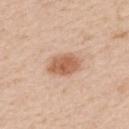workup=imaged on a skin check; not biopsied | location=the upper back | image=~15 mm tile from a whole-body skin photo | subject=female, aged 48 to 52 | illumination=white-light illumination | TBP lesion metrics=border irregularity of about 1.5 on a 0–10 scale and a within-lesion color-variation index near 3/10.